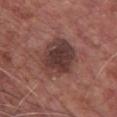• notes: total-body-photography surveillance lesion; no biopsy